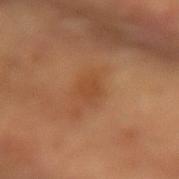notes — total-body-photography surveillance lesion; no biopsy
site — the right forearm
subject — female, aged around 55
image source — total-body-photography crop, ~15 mm field of view
illumination — cross-polarized illumination